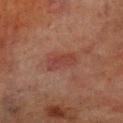biopsy status=catalogued during a skin exam; not biopsied
patient=male, roughly 70 years of age
imaging modality=~15 mm crop, total-body skin-cancer survey
tile lighting=cross-polarized
location=the leg
diameter=about 3.5 mm
TBP lesion metrics=a lesion area of about 6 mm², an eccentricity of roughly 0.85, and two-axis asymmetry of about 0.25; border irregularity of about 3 on a 0–10 scale and internal color variation of about 4 on a 0–10 scale